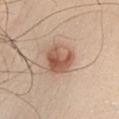follow-up = no biopsy performed (imaged during a skin exam).